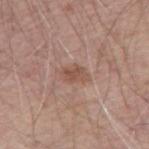follow-up = no biopsy performed (imaged during a skin exam)
lighting = white-light illumination
acquisition = total-body-photography crop, ~15 mm field of view
TBP lesion metrics = an area of roughly 4 mm², a shape eccentricity near 0.75, and a symmetry-axis asymmetry near 0.4; peripheral color asymmetry of about 0.5; a nevus-likeness score of about 0/100 and a lesion-detection confidence of about 100/100
body site = the right upper arm
patient = male, aged 68 to 72